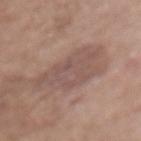Part of a total-body skin-imaging series; this lesion was reviewed on a skin check and was not flagged for biopsy. Cropped from a total-body skin-imaging series; the visible field is about 15 mm. Located on the chest. The subject is a female aged 73–77.